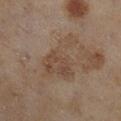Assessment: Captured during whole-body skin photography for melanoma surveillance; the lesion was not biopsied. Acquisition and patient details: Longest diameter approximately 6.5 mm. A 15 mm close-up tile from a total-body photography series done for melanoma screening. A female patient, aged 58 to 62. The tile uses cross-polarized illumination. On the leg. Automated tile analysis of the lesion measured a classifier nevus-likeness of about 0/100.